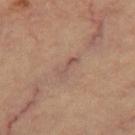A male subject approximately 60 years of age.
Measured at roughly 3 mm in maximum diameter.
Imaged with cross-polarized lighting.
Automated tile analysis of the lesion measured a footprint of about 3 mm², a shape eccentricity near 0.95, and two-axis asymmetry of about 0.55. The software also gave a nevus-likeness score of about 0/100.
On the leg.
A 15 mm close-up tile from a total-body photography series done for melanoma screening.